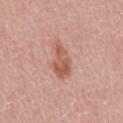follow-up: imaged on a skin check; not biopsied
patient: male, approximately 50 years of age
imaging modality: total-body-photography crop, ~15 mm field of view
lesion size: about 4.5 mm
anatomic site: the abdomen